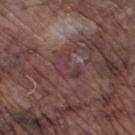Captured during whole-body skin photography for melanoma surveillance; the lesion was not biopsied. A male patient, aged approximately 75. The lesion-visualizer software estimated a peripheral color-asymmetry measure near 1. This is a white-light tile. On the left lower leg. Longest diameter approximately 3.5 mm. A roughly 15 mm field-of-view crop from a total-body skin photograph.